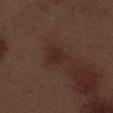workup: catalogued during a skin exam; not biopsied
image: ~15 mm crop, total-body skin-cancer survey
subject: male, aged 68 to 72
diameter: ~2.5 mm (longest diameter)
body site: the left thigh
image-analysis metrics: an area of roughly 5 mm², an outline eccentricity of about 0.45 (0 = round, 1 = elongated), and a shape-asymmetry score of about 0.2 (0 = symmetric); a mean CIELAB color near L≈26 a*≈18 b*≈20, about 5 CIELAB-L* units darker than the surrounding skin, and a normalized border contrast of about 6; border irregularity of about 1.5 on a 0–10 scale and radial color variation of about 0.5; a classifier nevus-likeness of about 15/100 and a lesion-detection confidence of about 100/100
illumination: white-light illumination